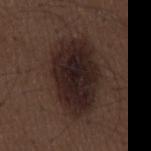workup — no biopsy performed (imaged during a skin exam)
illumination — white-light illumination
TBP lesion metrics — a lesion area of about 33 mm², an eccentricity of roughly 0.8, and a shape-asymmetry score of about 0.15 (0 = symmetric); a lesion–skin lightness drop of about 11 and a normalized border contrast of about 13; a border-irregularity rating of about 2/10, internal color variation of about 4.5 on a 0–10 scale, and peripheral color asymmetry of about 1; a classifier nevus-likeness of about 75/100 and lesion-presence confidence of about 100/100
size — ~8.5 mm (longest diameter)
acquisition — 15 mm crop, total-body photography
patient — male, approximately 50 years of age
site — the mid back